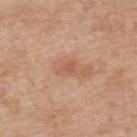Notes:
• subject: male, approximately 55 years of age
• acquisition: total-body-photography crop, ~15 mm field of view
• size: ~2.5 mm (longest diameter)
• location: the upper back